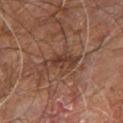Cropped from a total-body skin-imaging series; the visible field is about 15 mm.
Located on the right leg.
This is a cross-polarized tile.
The lesion's longest dimension is about 4.5 mm.
A male subject roughly 60 years of age.